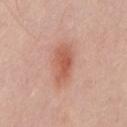Q: Was a biopsy performed?
A: catalogued during a skin exam; not biopsied
Q: What is the anatomic site?
A: the back
Q: Who is the patient?
A: male, in their mid-40s
Q: How was this image acquired?
A: ~15 mm crop, total-body skin-cancer survey